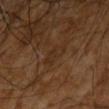Part of a total-body skin-imaging series; this lesion was reviewed on a skin check and was not flagged for biopsy. A male subject, aged 58 to 62. The lesion is on the right arm. A 15 mm close-up extracted from a 3D total-body photography capture.